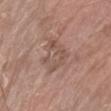Q: Was this lesion biopsied?
A: imaged on a skin check; not biopsied
Q: Automated lesion metrics?
A: border irregularity of about 4 on a 0–10 scale, a color-variation rating of about 4.5/10, and a peripheral color-asymmetry measure near 1.5; a nevus-likeness score of about 0/100 and lesion-presence confidence of about 85/100
Q: What is the anatomic site?
A: the arm
Q: What is the imaging modality?
A: ~15 mm tile from a whole-body skin photo
Q: Who is the patient?
A: female, about 75 years old
Q: How was the tile lit?
A: white-light illumination